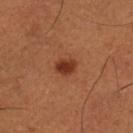<lesion>
  <biopsy_status>not biopsied; imaged during a skin examination</biopsy_status>
  <image>
    <source>total-body photography crop</source>
    <field_of_view_mm>15</field_of_view_mm>
  </image>
  <site>left lower leg</site>
  <lesion_size>
    <long_diameter_mm_approx>2.5</long_diameter_mm_approx>
  </lesion_size>
  <lighting>cross-polarized</lighting>
  <patient>
    <sex>male</sex>
    <age_approx>50</age_approx>
  </patient>
  <automated_metrics>
    <area_mm2_approx>4.0</area_mm2_approx>
    <cielab_L>36</cielab_L>
    <cielab_a>27</cielab_a>
    <cielab_b>32</cielab_b>
    <vs_skin_darker_L>12.0</vs_skin_darker_L>
    <vs_skin_contrast_norm>9.5</vs_skin_contrast_norm>
    <border_irregularity_0_10>1.0</border_irregularity_0_10>
    <color_variation_0_10>3.0</color_variation_0_10>
    <peripheral_color_asymmetry>1.0</peripheral_color_asymmetry>
  </automated_metrics>
</lesion>